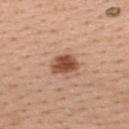Acquisition and patient details: A 15 mm crop from a total-body photograph taken for skin-cancer surveillance. The tile uses white-light illumination. The patient is a female aged approximately 40. Approximately 3.5 mm at its widest. From the upper back.